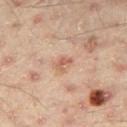Clinical impression: Recorded during total-body skin imaging; not selected for excision or biopsy. Background: Cropped from a total-body skin-imaging series; the visible field is about 15 mm. From the leg. An algorithmic analysis of the crop reported an automated nevus-likeness rating near 5 out of 100 and a detector confidence of about 100 out of 100 that the crop contains a lesion. The recorded lesion diameter is about 2 mm. Imaged with cross-polarized lighting. A male patient aged around 45.